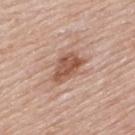Imaged during a routine full-body skin examination; the lesion was not biopsied and no histopathology is available.
A lesion tile, about 15 mm wide, cut from a 3D total-body photograph.
Automated tile analysis of the lesion measured an average lesion color of about L≈55 a*≈21 b*≈29 (CIELAB), roughly 12 lightness units darker than nearby skin, and a normalized border contrast of about 8.5. The software also gave border irregularity of about 3 on a 0–10 scale, internal color variation of about 3.5 on a 0–10 scale, and a peripheral color-asymmetry measure near 1.5.
A male subject aged 78 to 82.
The tile uses white-light illumination.
About 4.5 mm across.
The lesion is located on the upper back.